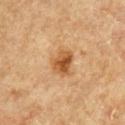Captured during whole-body skin photography for melanoma surveillance; the lesion was not biopsied. The subject is a male about 65 years old. Located on the chest. Cropped from a total-body skin-imaging series; the visible field is about 15 mm. Automated tile analysis of the lesion measured an area of roughly 7 mm², an eccentricity of roughly 0.65, and a shape-asymmetry score of about 0.3 (0 = symmetric). The analysis additionally found a lesion-to-skin contrast of about 9 (normalized; higher = more distinct). The analysis additionally found a color-variation rating of about 5/10 and a peripheral color-asymmetry measure near 1.5. It also reported a nevus-likeness score of about 85/100 and a detector confidence of about 100 out of 100 that the crop contains a lesion. Approximately 3.5 mm at its widest.